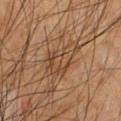| field | value |
|---|---|
| biopsy status | catalogued during a skin exam; not biopsied |
| subject | male, roughly 50 years of age |
| location | the chest |
| lesion size | about 5.5 mm |
| lighting | cross-polarized |
| image | total-body-photography crop, ~15 mm field of view |